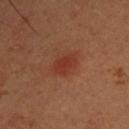The lesion was photographed on a routine skin check and not biopsied; there is no pathology result.
The recorded lesion diameter is about 3 mm.
Cropped from a whole-body photographic skin survey; the tile spans about 15 mm.
A male patient, aged 33–37.
Captured under cross-polarized illumination.
The lesion is located on the upper back.